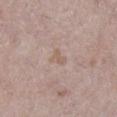  biopsy_status: not biopsied; imaged during a skin examination
  lesion_size:
    long_diameter_mm_approx: 2.5
  site: leg
  image:
    source: total-body photography crop
    field_of_view_mm: 15
  lighting: white-light
  patient:
    sex: female
    age_approx: 60
  automated_metrics:
    area_mm2_approx: 3.0
    eccentricity: 0.8
    shape_asymmetry: 0.55
    cielab_L: 59
    cielab_a: 16
    cielab_b: 25
    vs_skin_contrast_norm: 5.0
    border_irregularity_0_10: 5.0
    color_variation_0_10: 0.0
    nevus_likeness_0_100: 0
    lesion_detection_confidence_0_100: 100